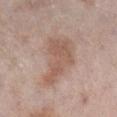Imaged during a routine full-body skin examination; the lesion was not biopsied and no histopathology is available.
From the right lower leg.
A male subject aged 58 to 62.
A lesion tile, about 15 mm wide, cut from a 3D total-body photograph.
Measured at roughly 6 mm in maximum diameter.
The tile uses white-light illumination.